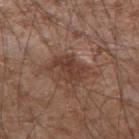patient:
  sex: male
  age_approx: 75
image:
  source: total-body photography crop
  field_of_view_mm: 15
lesion_size:
  long_diameter_mm_approx: 4.0
lighting: white-light
site: right upper arm